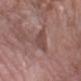| field | value |
|---|---|
| notes | imaged on a skin check; not biopsied |
| body site | the left forearm |
| acquisition | ~15 mm crop, total-body skin-cancer survey |
| subject | female, approximately 65 years of age |